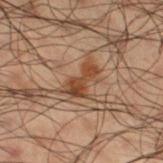Assessment:
The lesion was photographed on a routine skin check and not biopsied; there is no pathology result.
Acquisition and patient details:
This is a cross-polarized tile. A close-up tile cropped from a whole-body skin photograph, about 15 mm across. From the right thigh. A male subject, aged approximately 50.